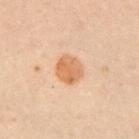Imaged during a routine full-body skin examination; the lesion was not biopsied and no histopathology is available.
This is a cross-polarized tile.
The recorded lesion diameter is about 3 mm.
A female patient approximately 30 years of age.
On the left upper arm.
A 15 mm close-up extracted from a 3D total-body photography capture.